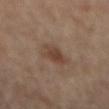Clinical impression: Captured during whole-body skin photography for melanoma surveillance; the lesion was not biopsied. Image and clinical context: Located on the back. A male subject roughly 65 years of age. Imaged with cross-polarized lighting. An algorithmic analysis of the crop reported a color-variation rating of about 3/10 and radial color variation of about 1. The software also gave an automated nevus-likeness rating near 70 out of 100 and a lesion-detection confidence of about 100/100. A 15 mm close-up extracted from a 3D total-body photography capture.